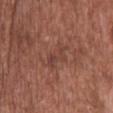Impression:
Part of a total-body skin-imaging series; this lesion was reviewed on a skin check and was not flagged for biopsy.
Image and clinical context:
Measured at roughly 4 mm in maximum diameter. Located on the upper back. This is a white-light tile. An algorithmic analysis of the crop reported a mean CIELAB color near L≈42 a*≈23 b*≈25 and about 6 CIELAB-L* units darker than the surrounding skin. The analysis additionally found an automated nevus-likeness rating near 0 out of 100 and a lesion-detection confidence of about 100/100. A lesion tile, about 15 mm wide, cut from a 3D total-body photograph. A male subject aged 43–47.